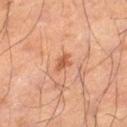{
  "biopsy_status": "not biopsied; imaged during a skin examination",
  "site": "left thigh",
  "lesion_size": {
    "long_diameter_mm_approx": 2.5
  },
  "automated_metrics": {
    "area_mm2_approx": 3.0,
    "eccentricity": 0.7,
    "border_irregularity_0_10": 3.5,
    "color_variation_0_10": 1.5,
    "peripheral_color_asymmetry": 0.5,
    "nevus_likeness_0_100": 75,
    "lesion_detection_confidence_0_100": 100
  },
  "patient": {
    "sex": "male",
    "age_approx": 55
  },
  "lighting": "cross-polarized",
  "image": {
    "source": "total-body photography crop",
    "field_of_view_mm": 15
  }
}